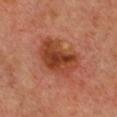workup = total-body-photography surveillance lesion; no biopsy
illumination = cross-polarized illumination
subject = female, about 40 years old
lesion diameter = ≈6 mm
image = ~15 mm crop, total-body skin-cancer survey
location = the chest